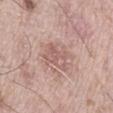Captured during whole-body skin photography for melanoma surveillance; the lesion was not biopsied.
Approximately 4 mm at its widest.
From the left thigh.
Automated image analysis of the tile measured a mean CIELAB color near L≈59 a*≈20 b*≈23, roughly 8 lightness units darker than nearby skin, and a normalized lesion–skin contrast near 5. The software also gave a border-irregularity index near 3/10 and radial color variation of about 1.5.
Captured under white-light illumination.
A region of skin cropped from a whole-body photographic capture, roughly 15 mm wide.
A male subject, aged 68 to 72.